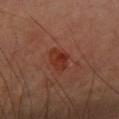Notes:
* notes · imaged on a skin check; not biopsied
* lesion size · ≈3.5 mm
* acquisition · total-body-photography crop, ~15 mm field of view
* anatomic site · the right forearm
* patient · male, aged around 40